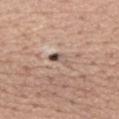{"biopsy_status": "not biopsied; imaged during a skin examination", "lighting": "white-light", "lesion_size": {"long_diameter_mm_approx": 3.5}, "site": "mid back", "automated_metrics": {"border_irregularity_0_10": 5.0, "color_variation_0_10": 5.5, "peripheral_color_asymmetry": 1.0, "nevus_likeness_0_100": 10}, "image": {"source": "total-body photography crop", "field_of_view_mm": 15}, "patient": {"sex": "male", "age_approx": 60}}